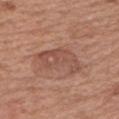This lesion was catalogued during total-body skin photography and was not selected for biopsy.